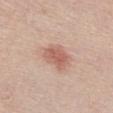Impression:
Imaged during a routine full-body skin examination; the lesion was not biopsied and no histopathology is available.
Context:
A 15 mm close-up tile from a total-body photography series done for melanoma screening. A female patient, aged 73–77. From the chest.